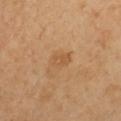workup: total-body-photography surveillance lesion; no biopsy
subject: female, in their 40s
anatomic site: the front of the torso
illumination: cross-polarized
automated metrics: a lesion color around L≈56 a*≈20 b*≈39 in CIELAB and a lesion–skin lightness drop of about 6; a nevus-likeness score of about 0/100 and lesion-presence confidence of about 100/100
image source: total-body-photography crop, ~15 mm field of view
lesion diameter: ~2.5 mm (longest diameter)